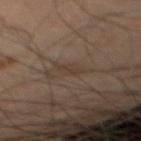Findings:
* workup: total-body-photography surveillance lesion; no biopsy
* automated metrics: an average lesion color of about L≈36 a*≈13 b*≈23 (CIELAB) and a lesion–skin lightness drop of about 5; a classifier nevus-likeness of about 0/100 and a lesion-detection confidence of about 90/100
* image source: total-body-photography crop, ~15 mm field of view
* anatomic site: the right lower leg
* lesion diameter: ≈2.5 mm
* patient: male, in their 70s
* tile lighting: cross-polarized